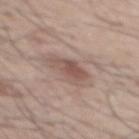Clinical impression: No biopsy was performed on this lesion — it was imaged during a full skin examination and was not determined to be concerning. Context: This is a white-light tile. Automated image analysis of the tile measured an area of roughly 7.5 mm², a shape eccentricity near 0.8, and a symmetry-axis asymmetry near 0.3. Located on the mid back. A region of skin cropped from a whole-body photographic capture, roughly 15 mm wide. A male patient aged 53 to 57.